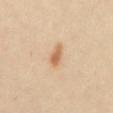follow-up: catalogued during a skin exam; not biopsied
image source: 15 mm crop, total-body photography
diameter: about 3 mm
TBP lesion metrics: a border-irregularity rating of about 2.5/10, internal color variation of about 2.5 on a 0–10 scale, and radial color variation of about 1; an automated nevus-likeness rating near 100 out of 100 and lesion-presence confidence of about 100/100
subject: female, aged 38–42
illumination: cross-polarized
anatomic site: the mid back